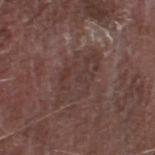follow-up = total-body-photography surveillance lesion; no biopsy